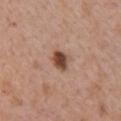The lesion was tiled from a total-body skin photograph and was not biopsied. An algorithmic analysis of the crop reported a lesion area of about 5 mm². The software also gave a mean CIELAB color near L≈47 a*≈21 b*≈28 and about 15 CIELAB-L* units darker than the surrounding skin. The software also gave a border-irregularity index near 2/10, a within-lesion color-variation index near 4.5/10, and a peripheral color-asymmetry measure near 1.5. A female patient in their 40s. Longest diameter approximately 2.5 mm. The tile uses white-light illumination. From the right upper arm. A 15 mm close-up tile from a total-body photography series done for melanoma screening.